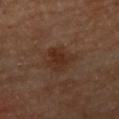– follow-up · catalogued during a skin exam; not biopsied
– image · ~15 mm crop, total-body skin-cancer survey
– TBP lesion metrics · a mean CIELAB color near L≈29 a*≈19 b*≈26, roughly 7 lightness units darker than nearby skin, and a normalized lesion–skin contrast near 7.5; a border-irregularity rating of about 3.5/10, a color-variation rating of about 3/10, and a peripheral color-asymmetry measure near 1; a nevus-likeness score of about 50/100 and lesion-presence confidence of about 100/100
– subject · male, aged 63 to 67
– location · the arm
– tile lighting · cross-polarized